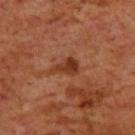<record>
<biopsy_status>not biopsied; imaged during a skin examination</biopsy_status>
<patient>
  <sex>male</sex>
  <age_approx>70</age_approx>
</patient>
<image>
  <source>total-body photography crop</source>
  <field_of_view_mm>15</field_of_view_mm>
</image>
<site>upper back</site>
</record>